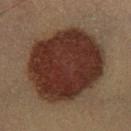biopsy status: total-body-photography surveillance lesion; no biopsy | subject: male, in their 40s | acquisition: ~15 mm crop, total-body skin-cancer survey | site: the left lower leg.